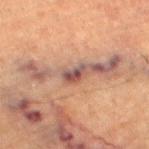– workup · total-body-photography surveillance lesion; no biopsy
– location · the left thigh
– subject · male, aged 83 or over
– automated lesion analysis · a lesion area of about 3 mm², an outline eccentricity of about 0.85 (0 = round, 1 = elongated), and a shape-asymmetry score of about 0.5 (0 = symmetric); a lesion color around L≈42 a*≈20 b*≈21 in CIELAB, a lesion–skin lightness drop of about 13, and a lesion-to-skin contrast of about 11.5 (normalized; higher = more distinct); a border-irregularity index near 4.5/10; a nevus-likeness score of about 0/100 and a detector confidence of about 55 out of 100 that the crop contains a lesion
– illumination · cross-polarized
– acquisition · ~15 mm crop, total-body skin-cancer survey